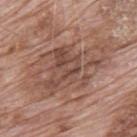<lesion>
  <biopsy_status>not biopsied; imaged during a skin examination</biopsy_status>
  <patient>
    <sex>male</sex>
    <age_approx>70</age_approx>
  </patient>
  <image>
    <source>total-body photography crop</source>
    <field_of_view_mm>15</field_of_view_mm>
  </image>
  <site>mid back</site>
</lesion>